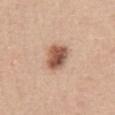Captured under white-light illumination.
Measured at roughly 3.5 mm in maximum diameter.
The lesion is located on the abdomen.
The lesion-visualizer software estimated an area of roughly 8.5 mm², an eccentricity of roughly 0.6, and a shape-asymmetry score of about 0.15 (0 = symmetric). It also reported a lesion color around L≈55 a*≈22 b*≈30 in CIELAB and about 16 CIELAB-L* units darker than the surrounding skin. The software also gave a border-irregularity index near 1.5/10, a color-variation rating of about 6.5/10, and peripheral color asymmetry of about 2. It also reported a lesion-detection confidence of about 100/100.
A 15 mm crop from a total-body photograph taken for skin-cancer surveillance.
A female subject, roughly 45 years of age.